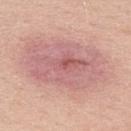Imaged during a routine full-body skin examination; the lesion was not biopsied and no histopathology is available. Located on the back. A male subject aged around 60. Cropped from a whole-body photographic skin survey; the tile spans about 15 mm. Automated image analysis of the tile measured a lesion area of about 60 mm², an eccentricity of roughly 0.8, and a shape-asymmetry score of about 0.15 (0 = symmetric). The software also gave an average lesion color of about L≈64 a*≈23 b*≈23 (CIELAB), a lesion–skin lightness drop of about 9, and a normalized lesion–skin contrast near 6.5. It also reported an automated nevus-likeness rating near 0 out of 100 and a detector confidence of about 100 out of 100 that the crop contains a lesion.